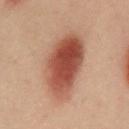Recorded during total-body skin imaging; not selected for excision or biopsy. A region of skin cropped from a whole-body photographic capture, roughly 15 mm wide. The lesion's longest dimension is about 8.5 mm. Automated tile analysis of the lesion measured an automated nevus-likeness rating near 100 out of 100 and lesion-presence confidence of about 100/100. On the front of the torso. A male subject, aged 38–42.